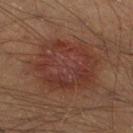Automated tile analysis of the lesion measured a border-irregularity index near 2.5/10, a within-lesion color-variation index near 4.5/10, and a peripheral color-asymmetry measure near 1.5. It also reported lesion-presence confidence of about 100/100. On the left lower leg. A roughly 15 mm field-of-view crop from a total-body skin photograph. The subject is a male aged 38 to 42.